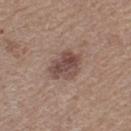Case summary:
- notes · total-body-photography surveillance lesion; no biopsy
- site · the left thigh
- acquisition · total-body-photography crop, ~15 mm field of view
- patient · female, aged approximately 55